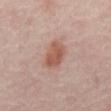Q: Is there a histopathology result?
A: catalogued during a skin exam; not biopsied
Q: Illumination type?
A: cross-polarized
Q: What kind of image is this?
A: total-body-photography crop, ~15 mm field of view
Q: Patient demographics?
A: aged 53 to 57
Q: What is the anatomic site?
A: the abdomen